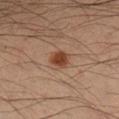Part of a total-body skin-imaging series; this lesion was reviewed on a skin check and was not flagged for biopsy. A lesion tile, about 15 mm wide, cut from a 3D total-body photograph. A male patient aged approximately 35. From the left forearm. This is a cross-polarized tile. About 2.5 mm across.